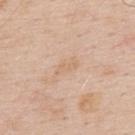Impression: Captured during whole-body skin photography for melanoma surveillance; the lesion was not biopsied. Context: On the upper back. A male patient about 60 years old. Automated image analysis of the tile measured a lesion area of about 3.5 mm², an outline eccentricity of about 0.9 (0 = round, 1 = elongated), and a symmetry-axis asymmetry near 0.3. The analysis additionally found an average lesion color of about L≈67 a*≈17 b*≈33 (CIELAB), about 5 CIELAB-L* units darker than the surrounding skin, and a normalized lesion–skin contrast near 4.5. And it measured a border-irregularity index near 3.5/10, a within-lesion color-variation index near 0/10, and a peripheral color-asymmetry measure near 0. Captured under white-light illumination. Approximately 3 mm at its widest. A close-up tile cropped from a whole-body skin photograph, about 15 mm across.